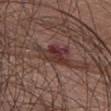| field | value |
|---|---|
| workup | imaged on a skin check; not biopsied |
| subject | female, roughly 50 years of age |
| image | ~15 mm crop, total-body skin-cancer survey |
| automated lesion analysis | a nevus-likeness score of about 0/100 and lesion-presence confidence of about 100/100 |
| site | the chest |
| lesion diameter | ~6 mm (longest diameter) |